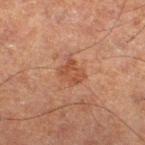  biopsy_status: not biopsied; imaged during a skin examination